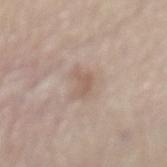Imaged during a routine full-body skin examination; the lesion was not biopsied and no histopathology is available.
Cropped from a whole-body photographic skin survey; the tile spans about 15 mm.
Located on the mid back.
The patient is a male about 80 years old.